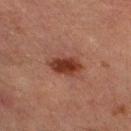<record>
  <lighting>cross-polarized</lighting>
  <patient>
    <sex>male</sex>
    <age_approx>85+</age_approx>
  </patient>
  <site>right lower leg</site>
  <image>
    <source>total-body photography crop</source>
    <field_of_view_mm>15</field_of_view_mm>
  </image>
  <lesion_size>
    <long_diameter_mm_approx>4.0</long_diameter_mm_approx>
  </lesion_size>
</record>